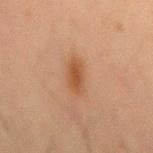  biopsy_status: not biopsied; imaged during a skin examination
  lesion_size:
    long_diameter_mm_approx: 4.0
  lighting: cross-polarized
  patient:
    sex: male
    age_approx: 70
  image:
    source: total-body photography crop
    field_of_view_mm: 15
  site: abdomen